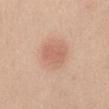Assessment:
Imaged during a routine full-body skin examination; the lesion was not biopsied and no histopathology is available.
Image and clinical context:
Measured at roughly 3.5 mm in maximum diameter. The lesion-visualizer software estimated a footprint of about 7.5 mm², a shape eccentricity near 0.65, and a symmetry-axis asymmetry near 0.2. The software also gave roughly 8 lightness units darker than nearby skin. The analysis additionally found internal color variation of about 2 on a 0–10 scale and peripheral color asymmetry of about 1. It also reported a nevus-likeness score of about 95/100 and a lesion-detection confidence of about 100/100. Cropped from a whole-body photographic skin survey; the tile spans about 15 mm. This is a white-light tile. The lesion is located on the abdomen. The subject is a female aged around 50.